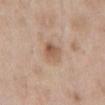notes: total-body-photography surveillance lesion; no biopsy | subject: male, approximately 60 years of age | diameter: ~3 mm (longest diameter) | body site: the front of the torso | image: ~15 mm crop, total-body skin-cancer survey.